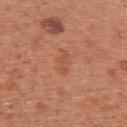  site: upper back
  lesion_size:
    long_diameter_mm_approx: 3.0
  automated_metrics:
    cielab_L: 53
    cielab_a: 26
    cielab_b: 34
    vs_skin_darker_L: 6.0
    vs_skin_contrast_norm: 4.5
    border_irregularity_0_10: 5.5
    color_variation_0_10: 0.0
    peripheral_color_asymmetry: 0.0
    nevus_likeness_0_100: 5
    lesion_detection_confidence_0_100: 100
  image:
    source: total-body photography crop
    field_of_view_mm: 15
  lighting: white-light
  patient:
    sex: female
    age_approx: 40